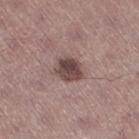{
  "biopsy_status": "not biopsied; imaged during a skin examination",
  "site": "right thigh",
  "patient": {
    "sex": "male",
    "age_approx": 45
  },
  "lighting": "white-light",
  "automated_metrics": {
    "nevus_likeness_0_100": 45,
    "lesion_detection_confidence_0_100": 100
  },
  "image": {
    "source": "total-body photography crop",
    "field_of_view_mm": 15
  }
}